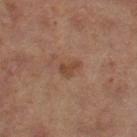follow-up — imaged on a skin check; not biopsied | diameter — ≈2.5 mm | lighting — cross-polarized | image — 15 mm crop, total-body photography | subject — female, about 60 years old | site — the leg.